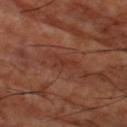image — ~15 mm crop, total-body skin-cancer survey
body site — the left thigh
patient — aged approximately 65
illumination — cross-polarized